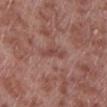Recorded during total-body skin imaging; not selected for excision or biopsy. The lesion is located on the left lower leg. Longest diameter approximately 3 mm. A male patient, aged approximately 55. The total-body-photography lesion software estimated an area of roughly 2.5 mm², a shape eccentricity near 0.95, and two-axis asymmetry of about 0.55. And it measured roughly 8 lightness units darker than nearby skin and a normalized lesion–skin contrast near 6. And it measured a border-irregularity index near 6.5/10 and peripheral color asymmetry of about 0. And it measured an automated nevus-likeness rating near 0 out of 100 and lesion-presence confidence of about 90/100. A 15 mm close-up tile from a total-body photography series done for melanoma screening.